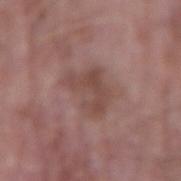  biopsy_status: not biopsied; imaged during a skin examination
  image:
    source: total-body photography crop
    field_of_view_mm: 15
  automated_metrics:
    shape_asymmetry: 0.35
    nevus_likeness_0_100: 0
    lesion_detection_confidence_0_100: 100
  site: left forearm
  lighting: white-light
  patient:
    sex: male
    age_approx: 65
  lesion_size:
    long_diameter_mm_approx: 5.0